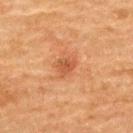Case summary:
– biopsy status · imaged on a skin check; not biopsied
– patient · female, about 70 years old
– image-analysis metrics · a lesion color around L≈46 a*≈25 b*≈35 in CIELAB, about 8 CIELAB-L* units darker than the surrounding skin, and a normalized lesion–skin contrast near 6.5; peripheral color asymmetry of about 1; a classifier nevus-likeness of about 25/100 and a lesion-detection confidence of about 100/100
– tile lighting · cross-polarized illumination
– image source · total-body-photography crop, ~15 mm field of view
– site · the upper back